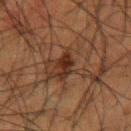Clinical impression: Recorded during total-body skin imaging; not selected for excision or biopsy. Context: A male patient, roughly 50 years of age. A 15 mm close-up tile from a total-body photography series done for melanoma screening. The lesion is located on the arm.